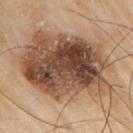Q: Was this lesion biopsied?
A: imaged on a skin check; not biopsied
Q: Who is the patient?
A: male, aged 63 to 67
Q: Where on the body is the lesion?
A: the arm
Q: How was this image acquired?
A: 15 mm crop, total-body photography
Q: What is the lesion's diameter?
A: ~14 mm (longest diameter)
Q: What lighting was used for the tile?
A: cross-polarized illumination